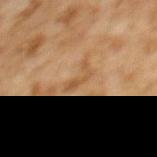No biopsy was performed on this lesion — it was imaged during a full skin examination and was not determined to be concerning. A 15 mm close-up tile from a total-body photography series done for melanoma screening. Measured at roughly 3.5 mm in maximum diameter. A female subject, aged 58–62. From the upper back.